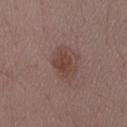Acquisition and patient details:
A male patient aged 48–52. Cropped from a total-body skin-imaging series; the visible field is about 15 mm. Automated tile analysis of the lesion measured a mean CIELAB color near L≈41 a*≈20 b*≈22, a lesion–skin lightness drop of about 8, and a normalized border contrast of about 7. The software also gave a classifier nevus-likeness of about 60/100 and a lesion-detection confidence of about 100/100. On the lower back.